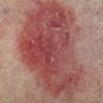follow-up — no biopsy performed (imaged during a skin exam); location — the leg; lesion diameter — ≈14 mm; imaging modality — ~15 mm tile from a whole-body skin photo; tile lighting — cross-polarized illumination; patient — male, aged around 75.